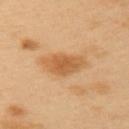follow-up=total-body-photography surveillance lesion; no biopsy
automated lesion analysis=a lesion area of about 11 mm² and an eccentricity of roughly 0.8; a border-irregularity index near 2.5/10 and radial color variation of about 1
tile lighting=cross-polarized
location=the upper back
diameter=about 5 mm
patient=male, aged approximately 40
image=15 mm crop, total-body photography